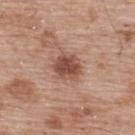workup: imaged on a skin check; not biopsied
anatomic site: the upper back
imaging modality: ~15 mm crop, total-body skin-cancer survey
size: ≈3.5 mm
patient: male, aged approximately 50